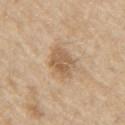workup = total-body-photography surveillance lesion; no biopsy | image = total-body-photography crop, ~15 mm field of view | automated metrics = a footprint of about 8 mm², a shape eccentricity near 0.65, and two-axis asymmetry of about 0.15; a lesion color around L≈58 a*≈16 b*≈34 in CIELAB, roughly 10 lightness units darker than nearby skin, and a normalized border contrast of about 7 | location = the right upper arm | subject = male, approximately 70 years of age.